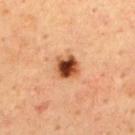biopsy_status: not biopsied; imaged during a skin examination
site: back
patient:
  sex: male
  age_approx: 65
image:
  source: total-body photography crop
  field_of_view_mm: 15
automated_metrics:
  area_mm2_approx: 6.5
  eccentricity: 0.25
  lesion_detection_confidence_0_100: 100
lesion_size:
  long_diameter_mm_approx: 3.0